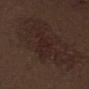Q: Was this lesion biopsied?
A: no biopsy performed (imaged during a skin exam)
Q: How large is the lesion?
A: about 6 mm
Q: What is the anatomic site?
A: the front of the torso
Q: What is the imaging modality?
A: total-body-photography crop, ~15 mm field of view
Q: What are the patient's age and sex?
A: male, aged 68–72